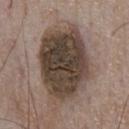Clinical impression: Part of a total-body skin-imaging series; this lesion was reviewed on a skin check and was not flagged for biopsy. Clinical summary: A male subject, approximately 75 years of age. About 10.5 mm across. The total-body-photography lesion software estimated a border-irregularity rating of about 2.5/10, a within-lesion color-variation index near 6.5/10, and peripheral color asymmetry of about 2. The software also gave an automated nevus-likeness rating near 5 out of 100 and a detector confidence of about 100 out of 100 that the crop contains a lesion. A 15 mm crop from a total-body photograph taken for skin-cancer surveillance. Located on the front of the torso.